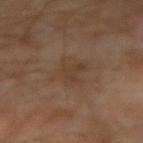biopsy status: imaged on a skin check; not biopsied | subject: male, in their 70s | image source: total-body-photography crop, ~15 mm field of view | location: the right forearm | automated metrics: a lesion area of about 9.5 mm² and an outline eccentricity of about 0.45 (0 = round, 1 = elongated); a border-irregularity rating of about 3.5/10, internal color variation of about 2.5 on a 0–10 scale, and a peripheral color-asymmetry measure near 1 | illumination: cross-polarized illumination | lesion diameter: ≈3.5 mm.